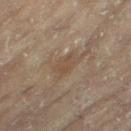Notes:
• follow-up · imaged on a skin check; not biopsied
• lesion size · ≈3.5 mm
• site · the right leg
• image · total-body-photography crop, ~15 mm field of view
• subject · female, roughly 80 years of age
• illumination · cross-polarized illumination